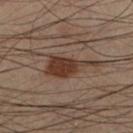Q: Is there a histopathology result?
A: no biopsy performed (imaged during a skin exam)
Q: What lighting was used for the tile?
A: cross-polarized illumination
Q: Lesion location?
A: the leg
Q: Lesion size?
A: about 6.5 mm
Q: What is the imaging modality?
A: total-body-photography crop, ~15 mm field of view
Q: What are the patient's age and sex?
A: male, aged 53–57